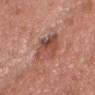biopsy status=total-body-photography surveillance lesion; no biopsy
imaging modality=~15 mm crop, total-body skin-cancer survey
site=the front of the torso
patient=male, aged around 55
tile lighting=white-light illumination